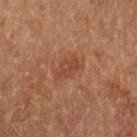Part of a total-body skin-imaging series; this lesion was reviewed on a skin check and was not flagged for biopsy. The lesion's longest dimension is about 3 mm. A 15 mm close-up extracted from a 3D total-body photography capture. The lesion is located on the left forearm. A female subject aged 68–72.